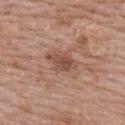The lesion was photographed on a routine skin check and not biopsied; there is no pathology result.
A female subject aged around 50.
On the upper back.
A close-up tile cropped from a whole-body skin photograph, about 15 mm across.